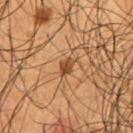This lesion was catalogued during total-body skin photography and was not selected for biopsy. Located on the front of the torso. A region of skin cropped from a whole-body photographic capture, roughly 15 mm wide. Longest diameter approximately 3 mm. A male subject, in their mid-50s. The total-body-photography lesion software estimated a footprint of about 3.5 mm² and a shape-asymmetry score of about 0.35 (0 = symmetric). It also reported an average lesion color of about L≈44 a*≈20 b*≈34 (CIELAB), about 11 CIELAB-L* units darker than the surrounding skin, and a normalized border contrast of about 8. And it measured a border-irregularity rating of about 3/10, internal color variation of about 2 on a 0–10 scale, and peripheral color asymmetry of about 1. The software also gave a lesion-detection confidence of about 100/100.